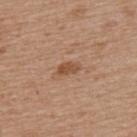| field | value |
|---|---|
| workup | imaged on a skin check; not biopsied |
| automated lesion analysis | a lesion color around L≈50 a*≈21 b*≈33 in CIELAB and about 10 CIELAB-L* units darker than the surrounding skin; a border-irregularity rating of about 3/10, internal color variation of about 1 on a 0–10 scale, and a peripheral color-asymmetry measure near 0 |
| anatomic site | the upper back |
| subject | female, aged 68–72 |
| lighting | white-light |
| image source | ~15 mm crop, total-body skin-cancer survey |